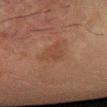Q: Is there a histopathology result?
A: catalogued during a skin exam; not biopsied
Q: How large is the lesion?
A: ≈3.5 mm
Q: Who is the patient?
A: male, approximately 60 years of age
Q: What is the imaging modality?
A: ~15 mm tile from a whole-body skin photo
Q: Automated lesion metrics?
A: border irregularity of about 3.5 on a 0–10 scale and a color-variation rating of about 1.5/10
Q: Where on the body is the lesion?
A: the left forearm